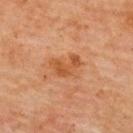{"biopsy_status": "not biopsied; imaged during a skin examination", "image": {"source": "total-body photography crop", "field_of_view_mm": 15}, "automated_metrics": {"cielab_L": 47, "cielab_a": 24, "cielab_b": 36, "vs_skin_contrast_norm": 7.0}, "patient": {"sex": "female", "age_approx": 70}, "lighting": "cross-polarized", "site": "back"}